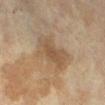workup=imaged on a skin check; not biopsied | illumination=cross-polarized illumination | lesion size=≈5.5 mm | body site=the right lower leg | image source=~15 mm tile from a whole-body skin photo | patient=female, aged 68 to 72.